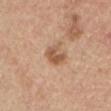• workup · imaged on a skin check; not biopsied
• patient · male, aged 68–72
• acquisition · 15 mm crop, total-body photography
• lighting · white-light illumination
• location · the mid back
• diameter · about 2.5 mm